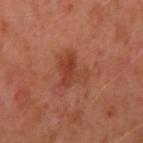– biopsy status — imaged on a skin check; not biopsied
– subject — female, aged approximately 40
– anatomic site — the right upper arm
– acquisition — ~15 mm crop, total-body skin-cancer survey
– TBP lesion metrics — an area of roughly 9 mm² and two-axis asymmetry of about 0.5; a mean CIELAB color near L≈42 a*≈28 b*≈33 and a lesion-to-skin contrast of about 6.5 (normalized; higher = more distinct); border irregularity of about 5.5 on a 0–10 scale, a within-lesion color-variation index near 4/10, and radial color variation of about 1.5
– size — about 4 mm
– tile lighting — cross-polarized illumination